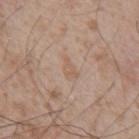Q: What are the patient's age and sex?
A: male, in their mid-50s
Q: Where on the body is the lesion?
A: the chest
Q: What lighting was used for the tile?
A: white-light
Q: What is the imaging modality?
A: total-body-photography crop, ~15 mm field of view
Q: How large is the lesion?
A: ≈3 mm